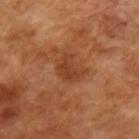biopsy status — imaged on a skin check; not biopsied | illumination — cross-polarized | image — total-body-photography crop, ~15 mm field of view | size — about 3 mm | patient — male, in their mid-60s.